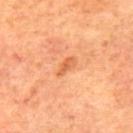About 3 mm across.
Located on the upper back.
A male patient, aged 68 to 72.
Automated tile analysis of the lesion measured an area of roughly 3.5 mm² and a shape-asymmetry score of about 0.3 (0 = symmetric). And it measured a mean CIELAB color near L≈61 a*≈30 b*≈43, about 9 CIELAB-L* units darker than the surrounding skin, and a normalized lesion–skin contrast near 6. The analysis additionally found a border-irregularity index near 3/10 and internal color variation of about 2 on a 0–10 scale.
A 15 mm close-up extracted from a 3D total-body photography capture.